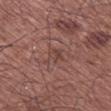{"biopsy_status": "not biopsied; imaged during a skin examination", "image": {"source": "total-body photography crop", "field_of_view_mm": 15}, "patient": {"sex": "male", "age_approx": 65}, "lesion_size": {"long_diameter_mm_approx": 2.5}, "automated_metrics": {"area_mm2_approx": 3.5, "eccentricity": 0.75, "cielab_L": 43, "cielab_a": 21, "cielab_b": 23, "vs_skin_contrast_norm": 5.0, "border_irregularity_0_10": 4.0, "color_variation_0_10": 2.5, "peripheral_color_asymmetry": 1.0, "nevus_likeness_0_100": 0, "lesion_detection_confidence_0_100": 70}, "site": "right lower leg"}